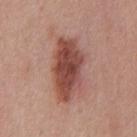Assessment: No biopsy was performed on this lesion — it was imaged during a full skin examination and was not determined to be concerning. Background: The subject is a male aged 28 to 32. Approximately 7.5 mm at its widest. A 15 mm close-up extracted from a 3D total-body photography capture. Located on the chest. An algorithmic analysis of the crop reported a border-irregularity index near 2.5/10 and peripheral color asymmetry of about 1.5. The analysis additionally found an automated nevus-likeness rating near 90 out of 100. The tile uses white-light illumination.